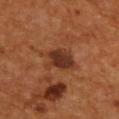Q: Is there a histopathology result?
A: imaged on a skin check; not biopsied